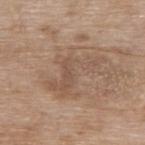The subject is a female about 75 years old. On the upper back. Cropped from a total-body skin-imaging series; the visible field is about 15 mm.